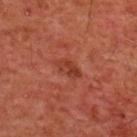Recorded during total-body skin imaging; not selected for excision or biopsy. The lesion is on the chest. Measured at roughly 3.5 mm in maximum diameter. A male subject aged around 60. This is a cross-polarized tile. A 15 mm close-up extracted from a 3D total-body photography capture.